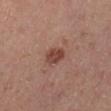This lesion was catalogued during total-body skin photography and was not selected for biopsy. The patient is a male roughly 55 years of age. The lesion's longest dimension is about 2.5 mm. The lesion is on the left lower leg. The total-body-photography lesion software estimated a lesion area of about 5 mm², a shape eccentricity near 0.75, and a shape-asymmetry score of about 0.2 (0 = symmetric). The analysis additionally found a border-irregularity index near 1.5/10 and radial color variation of about 1. Captured under cross-polarized illumination. A 15 mm close-up tile from a total-body photography series done for melanoma screening.